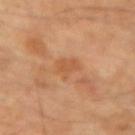Imaged during a routine full-body skin examination; the lesion was not biopsied and no histopathology is available. Cropped from a total-body skin-imaging series; the visible field is about 15 mm. The tile uses cross-polarized illumination. The lesion is on the left forearm. A female subject aged 58–62. About 3 mm across.